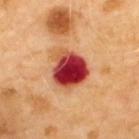  biopsy_status: not biopsied; imaged during a skin examination
  lighting: cross-polarized
  site: upper back
  image:
    source: total-body photography crop
    field_of_view_mm: 15
  automated_metrics:
    area_mm2_approx: 15.0
    eccentricity: 0.45
    shape_asymmetry: 0.25
    vs_skin_darker_L: 24.0
    vs_skin_contrast_norm: 16.0
  lesion_size:
    long_diameter_mm_approx: 4.5
  patient:
    sex: male
    age_approx: 70